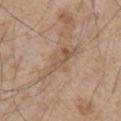Acquisition and patient details:
A male patient, aged approximately 65. Measured at roughly 6 mm in maximum diameter. A region of skin cropped from a whole-body photographic capture, roughly 15 mm wide. The lesion is on the chest.